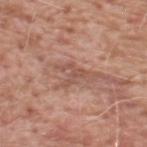notes: no biopsy performed (imaged during a skin exam) | subject: male, aged approximately 60 | anatomic site: the upper back | diameter: ≈2.5 mm | image source: ~15 mm crop, total-body skin-cancer survey | lighting: white-light.